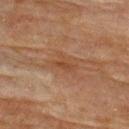No biopsy was performed on this lesion — it was imaged during a full skin examination and was not determined to be concerning. The subject is a female in their 80s. On the back. Cropped from a total-body skin-imaging series; the visible field is about 15 mm.